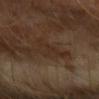biopsy_status: not biopsied; imaged during a skin examination
patient:
  sex: male
  age_approx: 60
lesion_size:
  long_diameter_mm_approx: 4.5
site: right forearm
lighting: cross-polarized
automated_metrics:
  area_mm2_approx: 6.5
  shape_asymmetry: 0.65
image:
  source: total-body photography crop
  field_of_view_mm: 15